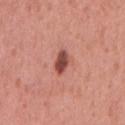biopsy_status: not biopsied; imaged during a skin examination
automated_metrics:
  area_mm2_approx: 4.5
  cielab_L: 48
  cielab_a: 27
  cielab_b: 27
  vs_skin_contrast_norm: 10.5
  border_irregularity_0_10: 2.5
  color_variation_0_10: 3.5
  peripheral_color_asymmetry: 1.0
  nevus_likeness_0_100: 90
  lesion_detection_confidence_0_100: 100
patient:
  sex: male
  age_approx: 55
lighting: white-light
site: mid back
image:
  source: total-body photography crop
  field_of_view_mm: 15